The tile uses white-light illumination, the lesion is on the left forearm, about 2 mm across, this image is a 15 mm lesion crop taken from a total-body photograph, the patient is a male roughly 55 years of age.
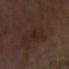{"diagnosis": {"histopathology": "actinic keratosis", "malignancy": "indeterminate", "taxonomic_path": ["Indeterminate", "Indeterminate epidermal proliferations", "Solar or actinic keratosis"]}}